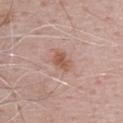Q: Where on the body is the lesion?
A: the head or neck
Q: What is the imaging modality?
A: 15 mm crop, total-body photography
Q: What are the patient's age and sex?
A: male, aged around 70
Q: What is the lesion's diameter?
A: ~2.5 mm (longest diameter)